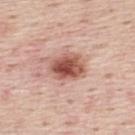This lesion was catalogued during total-body skin photography and was not selected for biopsy. Captured under white-light illumination. From the mid back. Approximately 4 mm at its widest. A 15 mm crop from a total-body photograph taken for skin-cancer surveillance. An algorithmic analysis of the crop reported an outline eccentricity of about 0.7 (0 = round, 1 = elongated) and a shape-asymmetry score of about 0.1 (0 = symmetric). The analysis additionally found an automated nevus-likeness rating near 95 out of 100 and a detector confidence of about 100 out of 100 that the crop contains a lesion. The patient is a male about 45 years old.